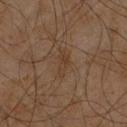<lesion>
  <biopsy_status>not biopsied; imaged during a skin examination</biopsy_status>
  <site>chest</site>
  <image>
    <source>total-body photography crop</source>
    <field_of_view_mm>15</field_of_view_mm>
  </image>
  <patient>
    <sex>male</sex>
    <age_approx>60</age_approx>
  </patient>
  <lighting>cross-polarized</lighting>
  <automated_metrics>
    <cielab_L>34</cielab_L>
    <cielab_a>15</cielab_a>
    <cielab_b>26</cielab_b>
    <vs_skin_darker_L>5.0</vs_skin_darker_L>
    <vs_skin_contrast_norm>5.5</vs_skin_contrast_norm>
    <nevus_likeness_0_100>0</nevus_likeness_0_100>
    <lesion_detection_confidence_0_100>100</lesion_detection_confidence_0_100>
  </automated_metrics>
  <lesion_size>
    <long_diameter_mm_approx>3.5</long_diameter_mm_approx>
  </lesion_size>
</lesion>